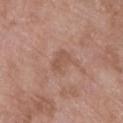Q: Was this lesion biopsied?
A: no biopsy performed (imaged during a skin exam)
Q: How was this image acquired?
A: 15 mm crop, total-body photography
Q: What is the anatomic site?
A: the left lower leg
Q: What lighting was used for the tile?
A: white-light illumination
Q: Patient demographics?
A: female, aged around 70